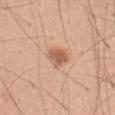Imaged during a routine full-body skin examination; the lesion was not biopsied and no histopathology is available.
Measured at roughly 2.5 mm in maximum diameter.
A male subject approximately 50 years of age.
A region of skin cropped from a whole-body photographic capture, roughly 15 mm wide.
Captured under white-light illumination.
The lesion is located on the abdomen.